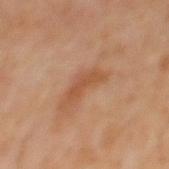Notes:
• biopsy status · no biopsy performed (imaged during a skin exam)
• body site · the mid back
• subject · male, aged 58–62
• lesion diameter · ≈3.5 mm
• automated metrics · border irregularity of about 4.5 on a 0–10 scale, internal color variation of about 1 on a 0–10 scale, and radial color variation of about 0; an automated nevus-likeness rating near 0 out of 100 and a detector confidence of about 100 out of 100 that the crop contains a lesion
• acquisition · ~15 mm crop, total-body skin-cancer survey